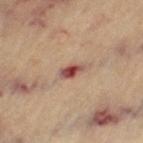The lesion was tiled from a total-body skin photograph and was not biopsied. The tile uses cross-polarized illumination. On the left thigh. A female subject, aged 63 to 67. The lesion's longest dimension is about 3 mm. A 15 mm close-up tile from a total-body photography series done for melanoma screening. The total-body-photography lesion software estimated an area of roughly 3.5 mm². And it measured a border-irregularity index near 2.5/10, internal color variation of about 6 on a 0–10 scale, and peripheral color asymmetry of about 1.5. The analysis additionally found a detector confidence of about 100 out of 100 that the crop contains a lesion.